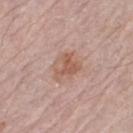  biopsy_status: not biopsied; imaged during a skin examination
  patient:
    sex: female
    age_approx: 65
  image:
    source: total-body photography crop
    field_of_view_mm: 15
  site: left thigh
  automated_metrics:
    area_mm2_approx: 7.5
    eccentricity: 0.5
    shape_asymmetry: 0.25
    vs_skin_darker_L: 8.0
    vs_skin_contrast_norm: 6.5
  lesion_size:
    long_diameter_mm_approx: 3.5
  lighting: white-light